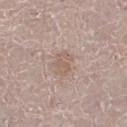The lesion was photographed on a routine skin check and not biopsied; there is no pathology result.
Imaged with white-light lighting.
Located on the left lower leg.
Longest diameter approximately 2.5 mm.
A female subject aged 53 to 57.
Cropped from a whole-body photographic skin survey; the tile spans about 15 mm.